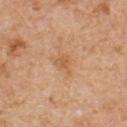Findings:
– notes · catalogued during a skin exam; not biopsied
– TBP lesion metrics · a lesion area of about 3.5 mm², an eccentricity of roughly 0.65, and a symmetry-axis asymmetry near 0.4; an average lesion color of about L≈59 a*≈22 b*≈38 (CIELAB), a lesion–skin lightness drop of about 7, and a lesion-to-skin contrast of about 5.5 (normalized; higher = more distinct); border irregularity of about 4 on a 0–10 scale; a nevus-likeness score of about 0/100 and lesion-presence confidence of about 100/100
– lesion diameter · ~2.5 mm (longest diameter)
– imaging modality · total-body-photography crop, ~15 mm field of view
– patient · male, about 55 years old
– lighting · white-light illumination
– body site · the chest